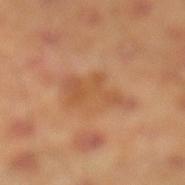biopsy status: imaged on a skin check; not biopsied | site: the leg | image source: ~15 mm crop, total-body skin-cancer survey | lesion diameter: ~5.5 mm (longest diameter) | subject: male, aged around 45 | lighting: cross-polarized illumination.